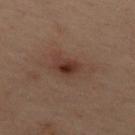Findings:
• notes — no biopsy performed (imaged during a skin exam)
• image — ~15 mm crop, total-body skin-cancer survey
• subject — female, in their mid- to late 50s
• lighting — cross-polarized
• location — the back